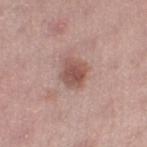workup: no biopsy performed (imaged during a skin exam) | tile lighting: white-light | anatomic site: the left lower leg | automated metrics: border irregularity of about 2 on a 0–10 scale, a within-lesion color-variation index near 3/10, and a peripheral color-asymmetry measure near 1 | subject: female, roughly 55 years of age | acquisition: ~15 mm crop, total-body skin-cancer survey | size: ≈3 mm.